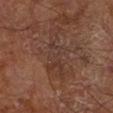No biopsy was performed on this lesion — it was imaged during a full skin examination and was not determined to be concerning. A male patient, in their mid- to late 60s. Captured under cross-polarized illumination. A region of skin cropped from a whole-body photographic capture, roughly 15 mm wide. Approximately 7 mm at its widest. The lesion-visualizer software estimated a lesion area of about 12 mm², a shape eccentricity near 0.95, and a symmetry-axis asymmetry near 0.55. The software also gave about 5 CIELAB-L* units darker than the surrounding skin. It also reported a color-variation rating of about 3.5/10 and peripheral color asymmetry of about 0.5. It also reported a classifier nevus-likeness of about 0/100 and lesion-presence confidence of about 80/100. Located on the left lower leg.